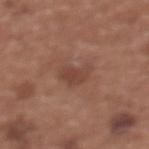Imaged during a routine full-body skin examination; the lesion was not biopsied and no histopathology is available.
A female subject in their mid- to late 30s.
A 15 mm close-up extracted from a 3D total-body photography capture.
The lesion is located on the chest.